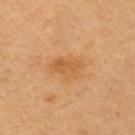| feature | finding |
|---|---|
| biopsy status | no biopsy performed (imaged during a skin exam) |
| subject | female, in their 40s |
| lesion diameter | ≈3.5 mm |
| automated lesion analysis | an area of roughly 6.5 mm², an eccentricity of roughly 0.7, and a shape-asymmetry score of about 0.3 (0 = symmetric) |
| location | the left upper arm |
| acquisition | 15 mm crop, total-body photography |
| illumination | cross-polarized illumination |